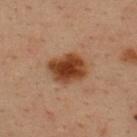Q: Is there a histopathology result?
A: no biopsy performed (imaged during a skin exam)
Q: How was the tile lit?
A: cross-polarized illumination
Q: What kind of image is this?
A: total-body-photography crop, ~15 mm field of view
Q: What is the anatomic site?
A: the upper back
Q: What is the lesion's diameter?
A: ≈4 mm
Q: Who is the patient?
A: male, aged 33–37